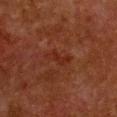notes: imaged on a skin check; not biopsied
image source: ~15 mm tile from a whole-body skin photo
patient: female, aged 48 to 52
site: the front of the torso
lighting: cross-polarized
lesion diameter: ~3.5 mm (longest diameter)